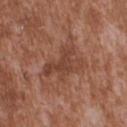Clinical impression:
The lesion was tiled from a total-body skin photograph and was not biopsied.
Acquisition and patient details:
The lesion's longest dimension is about 5 mm. A region of skin cropped from a whole-body photographic capture, roughly 15 mm wide. The lesion is located on the upper back. A male patient, approximately 45 years of age.